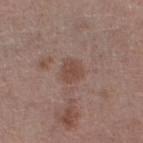Notes:
– workup: catalogued during a skin exam; not biopsied
– patient: male, aged around 65
– automated lesion analysis: an eccentricity of roughly 0.45 and a shape-asymmetry score of about 0.25 (0 = symmetric); a mean CIELAB color near L≈46 a*≈18 b*≈24, a lesion–skin lightness drop of about 7, and a normalized border contrast of about 6; lesion-presence confidence of about 100/100
– site: the left lower leg
– illumination: white-light
– image source: ~15 mm tile from a whole-body skin photo
– lesion diameter: ≈2.5 mm